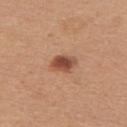Captured during whole-body skin photography for melanoma surveillance; the lesion was not biopsied.
A female patient aged 43 to 47.
Located on the upper back.
This image is a 15 mm lesion crop taken from a total-body photograph.